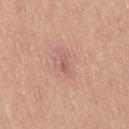  biopsy_status: not biopsied; imaged during a skin examination
  patient:
    sex: male
    age_approx: 30
  lighting: white-light
  lesion_size:
    long_diameter_mm_approx: 2.5
  image:
    source: total-body photography crop
    field_of_view_mm: 15
  site: right thigh